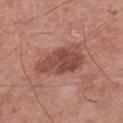Part of a total-body skin-imaging series; this lesion was reviewed on a skin check and was not flagged for biopsy. A male subject, in their mid-70s. Captured under white-light illumination. From the left lower leg. A lesion tile, about 15 mm wide, cut from a 3D total-body photograph.